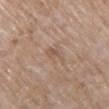Q: Was a biopsy performed?
A: catalogued during a skin exam; not biopsied
Q: Where on the body is the lesion?
A: the chest
Q: What is the imaging modality?
A: ~15 mm crop, total-body skin-cancer survey
Q: What lighting was used for the tile?
A: white-light illumination
Q: Lesion size?
A: ≈3 mm
Q: What did automated image analysis measure?
A: an eccentricity of roughly 0.9 and a shape-asymmetry score of about 0.45 (0 = symmetric)
Q: Who is the patient?
A: female, roughly 85 years of age or older